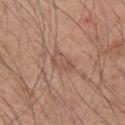No biopsy was performed on this lesion — it was imaged during a full skin examination and was not determined to be concerning. This image is a 15 mm lesion crop taken from a total-body photograph. Located on the right upper arm. Measured at roughly 3 mm in maximum diameter. The total-body-photography lesion software estimated an area of roughly 4.5 mm², a shape eccentricity near 0.8, and a symmetry-axis asymmetry near 0.3. The analysis additionally found border irregularity of about 3 on a 0–10 scale and peripheral color asymmetry of about 0.5. The patient is a male in their mid- to late 40s.